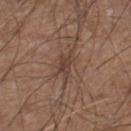Part of a total-body skin-imaging series; this lesion was reviewed on a skin check and was not flagged for biopsy.
This is a white-light tile.
An algorithmic analysis of the crop reported a lesion color around L≈41 a*≈16 b*≈25 in CIELAB and a normalized border contrast of about 6.5. And it measured radial color variation of about 1.
On the leg.
A lesion tile, about 15 mm wide, cut from a 3D total-body photograph.
A male patient approximately 60 years of age.
Approximately 4 mm at its widest.